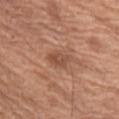Findings:
* follow-up — imaged on a skin check; not biopsied
* location — the abdomen
* image — total-body-photography crop, ~15 mm field of view
* subject — female, in their mid- to late 60s